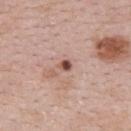Q: Was a biopsy performed?
A: catalogued during a skin exam; not biopsied
Q: How large is the lesion?
A: ~2 mm (longest diameter)
Q: Automated lesion metrics?
A: an average lesion color of about L≈51 a*≈22 b*≈26 (CIELAB) and a normalized lesion–skin contrast near 10.5
Q: Who is the patient?
A: female, aged around 30
Q: What lighting was used for the tile?
A: white-light illumination
Q: Lesion location?
A: the back
Q: How was this image acquired?
A: ~15 mm tile from a whole-body skin photo